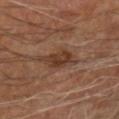Clinical impression:
Part of a total-body skin-imaging series; this lesion was reviewed on a skin check and was not flagged for biopsy.